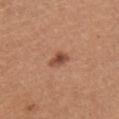acquisition: ~15 mm crop, total-body skin-cancer survey
lesion diameter: about 2.5 mm
anatomic site: the chest
subject: female, aged 23–27
automated lesion analysis: a lesion color around L≈49 a*≈24 b*≈32 in CIELAB, a lesion–skin lightness drop of about 12, and a lesion-to-skin contrast of about 8.5 (normalized; higher = more distinct); a color-variation rating of about 3.5/10 and a peripheral color-asymmetry measure near 1
illumination: white-light illumination